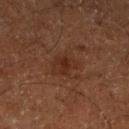{"biopsy_status": "not biopsied; imaged during a skin examination", "image": {"source": "total-body photography crop", "field_of_view_mm": 15}, "lighting": "cross-polarized", "patient": {"sex": "male", "age_approx": 65}, "automated_metrics": {"eccentricity": 0.7, "shape_asymmetry": 0.35, "border_irregularity_0_10": 3.5, "peripheral_color_asymmetry": 0.5, "nevus_likeness_0_100": 5}, "lesion_size": {"long_diameter_mm_approx": 4.0}, "site": "left lower leg"}